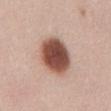Q: How was this image acquired?
A: 15 mm crop, total-body photography
Q: What is the anatomic site?
A: the front of the torso
Q: Patient demographics?
A: female, aged 38 to 42